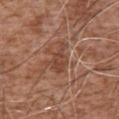• follow-up · total-body-photography surveillance lesion; no biopsy
• illumination · white-light
• TBP lesion metrics · a footprint of about 6.5 mm², a shape eccentricity near 0.8, and a shape-asymmetry score of about 0.45 (0 = symmetric); a nevus-likeness score of about 0/100 and lesion-presence confidence of about 95/100
• image · 15 mm crop, total-body photography
• body site · the chest
• diameter · ≈3.5 mm
• subject · male, aged 73 to 77